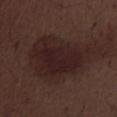The lesion was photographed on a routine skin check and not biopsied; there is no pathology result. A male subject, aged 68 to 72. The lesion is on the left lower leg. A 15 mm close-up extracted from a 3D total-body photography capture.